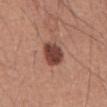subject: male, about 45 years old
imaging modality: ~15 mm tile from a whole-body skin photo
lesion size: about 3.5 mm
TBP lesion metrics: an outline eccentricity of about 0.55 (0 = round, 1 = elongated) and a shape-asymmetry score of about 0.15 (0 = symmetric); a border-irregularity rating of about 1.5/10 and a color-variation rating of about 3/10
anatomic site: the mid back